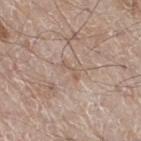Clinical summary: The tile uses white-light illumination. Cropped from a total-body skin-imaging series; the visible field is about 15 mm. The lesion is on the right thigh. Longest diameter approximately 2.5 mm. A male patient in their 60s. The total-body-photography lesion software estimated an eccentricity of roughly 0.95 and two-axis asymmetry of about 0.5. It also reported a lesion–skin lightness drop of about 6 and a normalized lesion–skin contrast near 4.5. The analysis additionally found a border-irregularity rating of about 5.5/10 and a peripheral color-asymmetry measure near 0. The analysis additionally found a classifier nevus-likeness of about 0/100 and a lesion-detection confidence of about 60/100.